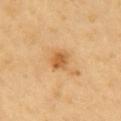<tbp_lesion>
  <biopsy_status>not biopsied; imaged during a skin examination</biopsy_status>
  <site>chest</site>
  <image>
    <source>total-body photography crop</source>
    <field_of_view_mm>15</field_of_view_mm>
  </image>
  <patient>
    <sex>male</sex>
    <age_approx>60</age_approx>
  </patient>
</tbp_lesion>